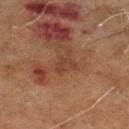No biopsy was performed on this lesion — it was imaged during a full skin examination and was not determined to be concerning.
A male patient, aged 68–72.
A 15 mm close-up tile from a total-body photography series done for melanoma screening.
From the left lower leg.
The tile uses cross-polarized illumination.
Automated image analysis of the tile measured a shape eccentricity near 0.7 and a shape-asymmetry score of about 0.55 (0 = symmetric). It also reported a mean CIELAB color near L≈33 a*≈19 b*≈24, roughly 5 lightness units darker than nearby skin, and a normalized border contrast of about 5. The software also gave a lesion-detection confidence of about 100/100.